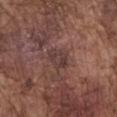Clinical impression:
The lesion was photographed on a routine skin check and not biopsied; there is no pathology result.
Acquisition and patient details:
An algorithmic analysis of the crop reported a classifier nevus-likeness of about 0/100 and a detector confidence of about 90 out of 100 that the crop contains a lesion. A male patient aged 73 to 77. From the chest. Cropped from a total-body skin-imaging series; the visible field is about 15 mm. The lesion's longest dimension is about 3 mm. Imaged with white-light lighting.